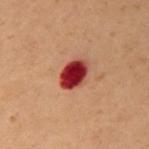Impression:
Imaged during a routine full-body skin examination; the lesion was not biopsied and no histopathology is available.
Image and clinical context:
The lesion-visualizer software estimated a shape eccentricity near 0.7. The software also gave border irregularity of about 1.5 on a 0–10 scale and a peripheral color-asymmetry measure near 1.5. Cropped from a total-body skin-imaging series; the visible field is about 15 mm. A female subject, aged approximately 60. Imaged with cross-polarized lighting. Longest diameter approximately 4 mm. The lesion is located on the chest.